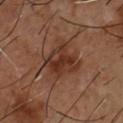Part of a total-body skin-imaging series; this lesion was reviewed on a skin check and was not flagged for biopsy.
A male subject in their mid-50s.
A close-up tile cropped from a whole-body skin photograph, about 15 mm across.
The lesion is located on the chest.